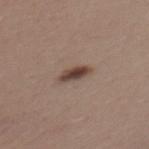  biopsy_status: not biopsied; imaged during a skin examination
  site: upper back
  lesion_size:
    long_diameter_mm_approx: 3.5
  lighting: white-light
  patient:
    sex: female
    age_approx: 25
  image:
    source: total-body photography crop
    field_of_view_mm: 15
  automated_metrics:
    area_mm2_approx: 4.5
    eccentricity: 0.85
    shape_asymmetry: 0.15
    cielab_L: 43
    cielab_a: 16
    cielab_b: 23
    vs_skin_contrast_norm: 10.5
    border_irregularity_0_10: 2.0
    color_variation_0_10: 3.5
    peripheral_color_asymmetry: 1.0
    nevus_likeness_0_100: 95
    lesion_detection_confidence_0_100: 100